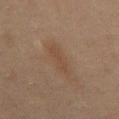Findings:
– biopsy status · imaged on a skin check; not biopsied
– body site · the back
– lighting · cross-polarized illumination
– image source · ~15 mm crop, total-body skin-cancer survey
– image-analysis metrics · a footprint of about 6 mm² and a symmetry-axis asymmetry near 0.35; a mean CIELAB color near L≈36 a*≈14 b*≈24; a border-irregularity index near 5/10 and a peripheral color-asymmetry measure near 0.5
– lesion diameter · ≈5 mm
– subject · male, approximately 45 years of age